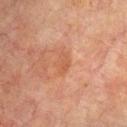Notes:
- biopsy status: no biopsy performed (imaged during a skin exam)
- lesion diameter: ~2.5 mm (longest diameter)
- automated lesion analysis: an average lesion color of about L≈45 a*≈22 b*≈30 (CIELAB), about 5 CIELAB-L* units darker than the surrounding skin, and a normalized lesion–skin contrast near 5; a classifier nevus-likeness of about 0/100 and lesion-presence confidence of about 100/100
- site: the upper back
- illumination: cross-polarized illumination
- subject: male, aged 63 to 67
- image source: total-body-photography crop, ~15 mm field of view